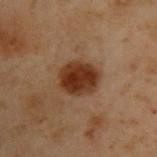Captured during whole-body skin photography for melanoma surveillance; the lesion was not biopsied.
From the back.
Cropped from a total-body skin-imaging series; the visible field is about 15 mm.
A male patient aged 53–57.